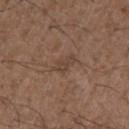Q: Was a biopsy performed?
A: catalogued during a skin exam; not biopsied
Q: What is the anatomic site?
A: the right upper arm
Q: What is the imaging modality?
A: 15 mm crop, total-body photography
Q: Who is the patient?
A: male, roughly 50 years of age